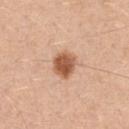workup — total-body-photography surveillance lesion; no biopsy | size — ~3.5 mm (longest diameter) | acquisition — ~15 mm crop, total-body skin-cancer survey | anatomic site — the front of the torso | subject — male, aged around 50 | tile lighting — white-light illumination.